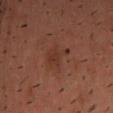Case summary:
- follow-up — total-body-photography surveillance lesion; no biopsy
- lesion size — ~3 mm (longest diameter)
- body site — the head or neck
- image — ~15 mm crop, total-body skin-cancer survey
- subject — male, approximately 40 years of age